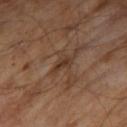follow-up: catalogued during a skin exam; not biopsied | subject: male, aged approximately 65 | TBP lesion metrics: a lesion color around L≈35 a*≈17 b*≈27 in CIELAB, roughly 7 lightness units darker than nearby skin, and a lesion-to-skin contrast of about 6.5 (normalized; higher = more distinct); a border-irregularity index near 4/10 and peripheral color asymmetry of about 0.5; an automated nevus-likeness rating near 0 out of 100 | imaging modality: 15 mm crop, total-body photography | illumination: cross-polarized illumination.